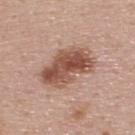No biopsy was performed on this lesion — it was imaged during a full skin examination and was not determined to be concerning.
A region of skin cropped from a whole-body photographic capture, roughly 15 mm wide.
Captured under white-light illumination.
From the upper back.
The patient is a male roughly 55 years of age.
The lesion's longest dimension is about 6 mm.